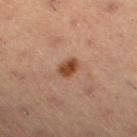Recorded during total-body skin imaging; not selected for excision or biopsy. The lesion is located on the left lower leg. A roughly 15 mm field-of-view crop from a total-body skin photograph. A male patient aged 53 to 57.